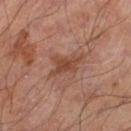Q: Was a biopsy performed?
A: catalogued during a skin exam; not biopsied
Q: How large is the lesion?
A: ≈3.5 mm
Q: Patient demographics?
A: male, aged 53 to 57
Q: How was this image acquired?
A: total-body-photography crop, ~15 mm field of view
Q: Illumination type?
A: cross-polarized illumination
Q: Where on the body is the lesion?
A: the leg